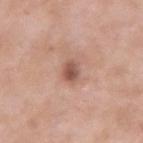Q: Is there a histopathology result?
A: total-body-photography surveillance lesion; no biopsy
Q: Who is the patient?
A: male, aged approximately 55
Q: What kind of image is this?
A: 15 mm crop, total-body photography
Q: Illumination type?
A: white-light illumination
Q: Where on the body is the lesion?
A: the arm